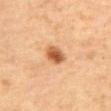The lesion was tiled from a total-body skin photograph and was not biopsied.
Located on the abdomen.
A female patient aged 58–62.
Measured at roughly 3 mm in maximum diameter.
Imaged with cross-polarized lighting.
Cropped from a whole-body photographic skin survey; the tile spans about 15 mm.
Automated tile analysis of the lesion measured an eccentricity of roughly 0.65 and two-axis asymmetry of about 0.15. It also reported an average lesion color of about L≈55 a*≈24 b*≈38 (CIELAB), about 14 CIELAB-L* units darker than the surrounding skin, and a normalized border contrast of about 9.5. The analysis additionally found a nevus-likeness score of about 100/100 and a lesion-detection confidence of about 100/100.